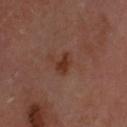biopsy status: no biopsy performed (imaged during a skin exam) | subject: aged 63 to 67 | image: ~15 mm crop, total-body skin-cancer survey | tile lighting: cross-polarized | lesion diameter: ≈2.5 mm | anatomic site: the head or neck.